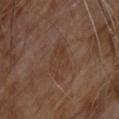Q: Was a biopsy performed?
A: total-body-photography surveillance lesion; no biopsy
Q: What is the anatomic site?
A: the upper back
Q: What kind of image is this?
A: 15 mm crop, total-body photography
Q: Who is the patient?
A: male, aged 68–72